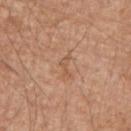workup: catalogued during a skin exam; not biopsied
automated lesion analysis: an area of roughly 2 mm² and a shape eccentricity near 0.9
size: ≈3 mm
imaging modality: 15 mm crop, total-body photography
tile lighting: white-light illumination
body site: the right upper arm
subject: male, aged 78–82